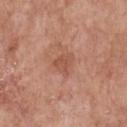Assessment: No biopsy was performed on this lesion — it was imaged during a full skin examination and was not determined to be concerning. Background: From the chest. Imaged with white-light lighting. The total-body-photography lesion software estimated an eccentricity of roughly 0.5 and a symmetry-axis asymmetry near 0.4. A 15 mm close-up tile from a total-body photography series done for melanoma screening. A female patient, about 60 years old. The recorded lesion diameter is about 3 mm.